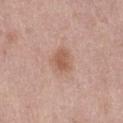| field | value |
|---|---|
| site | the abdomen |
| illumination | white-light |
| image | ~15 mm tile from a whole-body skin photo |
| patient | female, approximately 40 years of age |